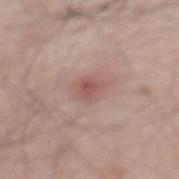The lesion-visualizer software estimated an automated nevus-likeness rating near 25 out of 100. A male patient, aged around 65. Longest diameter approximately 2.5 mm. On the back. Imaged with white-light lighting. A roughly 15 mm field-of-view crop from a total-body skin photograph.